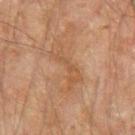Q: What kind of image is this?
A: total-body-photography crop, ~15 mm field of view
Q: What is the anatomic site?
A: the arm
Q: Patient demographics?
A: male, aged 43–47